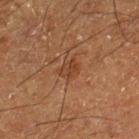The lesion was photographed on a routine skin check and not biopsied; there is no pathology result. The tile uses cross-polarized illumination. A close-up tile cropped from a whole-body skin photograph, about 15 mm across. Approximately 2.5 mm at its widest. Located on the right lower leg. A male subject, in their mid- to late 60s.